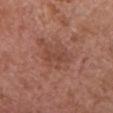Captured during whole-body skin photography for melanoma surveillance; the lesion was not biopsied.
Located on the chest.
This image is a 15 mm lesion crop taken from a total-body photograph.
The subject is a female aged 63–67.